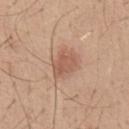• location: the abdomen
• illumination: white-light illumination
• acquisition: total-body-photography crop, ~15 mm field of view
• lesion size: about 3.5 mm
• patient: male, roughly 40 years of age